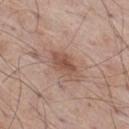location=the right thigh; patient=male, aged 53–57; imaging modality=~15 mm crop, total-body skin-cancer survey.